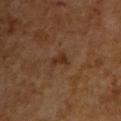notes: catalogued during a skin exam; not biopsied | subject: male, approximately 60 years of age | lesion diameter: ≈2.5 mm | automated metrics: a border-irregularity index near 4.5/10, a color-variation rating of about 1.5/10, and peripheral color asymmetry of about 0.5 | tile lighting: cross-polarized | image source: 15 mm crop, total-body photography | anatomic site: the upper back.